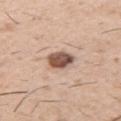size=about 3 mm | site=the left upper arm | patient=male, approximately 50 years of age | tile lighting=white-light illumination | image=~15 mm tile from a whole-body skin photo.